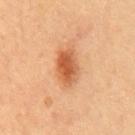Q: Was this lesion biopsied?
A: imaged on a skin check; not biopsied
Q: What kind of image is this?
A: ~15 mm tile from a whole-body skin photo
Q: Patient demographics?
A: female, in their mid-50s
Q: Lesion size?
A: about 4.5 mm
Q: What did automated image analysis measure?
A: a lesion area of about 9 mm² and a shape-asymmetry score of about 0.15 (0 = symmetric); a mean CIELAB color near L≈54 a*≈27 b*≈38, a lesion–skin lightness drop of about 13, and a normalized border contrast of about 8.5
Q: What is the anatomic site?
A: the front of the torso
Q: How was the tile lit?
A: cross-polarized illumination